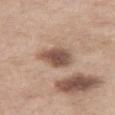Impression: Recorded during total-body skin imaging; not selected for excision or biopsy. Clinical summary: The lesion-visualizer software estimated roughly 14 lightness units darker than nearby skin and a normalized lesion–skin contrast near 10. It also reported a border-irregularity index near 2/10, a color-variation rating of about 4.5/10, and radial color variation of about 1. Cropped from a total-body skin-imaging series; the visible field is about 15 mm. Measured at roughly 4 mm in maximum diameter. The subject is a female about 55 years old. The lesion is on the leg. Captured under white-light illumination.